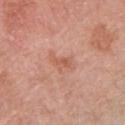biopsy_status: not biopsied; imaged during a skin examination
image:
  source: total-body photography crop
  field_of_view_mm: 15
site: arm
patient:
  sex: female
  age_approx: 60